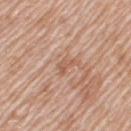This lesion was catalogued during total-body skin photography and was not selected for biopsy.
A 15 mm crop from a total-body photograph taken for skin-cancer surveillance.
Captured under white-light illumination.
Automated image analysis of the tile measured an area of roughly 2.5 mm², an outline eccentricity of about 0.85 (0 = round, 1 = elongated), and a shape-asymmetry score of about 0.5 (0 = symmetric). The analysis additionally found an average lesion color of about L≈57 a*≈21 b*≈31 (CIELAB), a lesion–skin lightness drop of about 7, and a normalized lesion–skin contrast near 5.5. The software also gave a lesion-detection confidence of about 90/100.
The subject is a female in their 70s.
The lesion's longest dimension is about 2.5 mm.
On the arm.